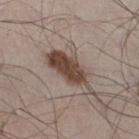Impression:
The lesion was tiled from a total-body skin photograph and was not biopsied.
Acquisition and patient details:
A close-up tile cropped from a whole-body skin photograph, about 15 mm across. An algorithmic analysis of the crop reported a lesion area of about 13 mm² and two-axis asymmetry of about 0.3. And it measured a color-variation rating of about 4/10 and peripheral color asymmetry of about 1.5. And it measured a classifier nevus-likeness of about 95/100. The patient is a male roughly 50 years of age. The lesion is on the right thigh. Measured at roughly 7.5 mm in maximum diameter.